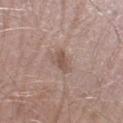Captured during whole-body skin photography for melanoma surveillance; the lesion was not biopsied.
A male subject in their mid- to late 40s.
Automated tile analysis of the lesion measured an automated nevus-likeness rating near 20 out of 100 and lesion-presence confidence of about 100/100.
A 15 mm close-up extracted from a 3D total-body photography capture.
Approximately 3 mm at its widest.
On the right lower leg.